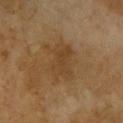workup=catalogued during a skin exam; not biopsied
subject=female, aged approximately 60
location=the upper back
acquisition=total-body-photography crop, ~15 mm field of view
tile lighting=cross-polarized illumination
size=≈4.5 mm